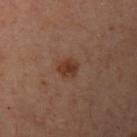• notes · no biopsy performed (imaged during a skin exam)
• lesion diameter · ≈2.5 mm
• image source · ~15 mm crop, total-body skin-cancer survey
• automated lesion analysis · an area of roughly 4.5 mm², an eccentricity of roughly 0.55, and two-axis asymmetry of about 0.15; a lesion color around L≈29 a*≈17 b*≈23 in CIELAB, a lesion–skin lightness drop of about 7, and a lesion-to-skin contrast of about 8 (normalized; higher = more distinct); a color-variation rating of about 2/10; a classifier nevus-likeness of about 85/100 and a detector confidence of about 100 out of 100 that the crop contains a lesion
• tile lighting · cross-polarized illumination
• anatomic site · the arm
• subject · female, about 55 years old